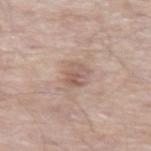The lesion was tiled from a total-body skin photograph and was not biopsied.
This is a white-light tile.
A male patient in their mid-60s.
Cropped from a whole-body photographic skin survey; the tile spans about 15 mm.
An algorithmic analysis of the crop reported an area of roughly 4.5 mm², a shape eccentricity near 0.75, and two-axis asymmetry of about 0.3.
The lesion's longest dimension is about 3 mm.
From the leg.